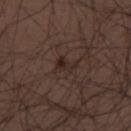The lesion was photographed on a routine skin check and not biopsied; there is no pathology result. Captured under white-light illumination. An algorithmic analysis of the crop reported an area of roughly 3.5 mm² and an outline eccentricity of about 0.8 (0 = round, 1 = elongated). And it measured a lesion color around L≈26 a*≈13 b*≈19 in CIELAB, about 6 CIELAB-L* units darker than the surrounding skin, and a normalized lesion–skin contrast near 6.5. The software also gave border irregularity of about 4 on a 0–10 scale and a within-lesion color-variation index near 1.5/10. The analysis additionally found a classifier nevus-likeness of about 35/100 and a detector confidence of about 100 out of 100 that the crop contains a lesion. A male subject approximately 30 years of age. Cropped from a whole-body photographic skin survey; the tile spans about 15 mm. The lesion is on the back.